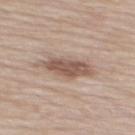Q: Was this lesion biopsied?
A: total-body-photography surveillance lesion; no biopsy
Q: Automated lesion metrics?
A: a lesion–skin lightness drop of about 12 and a normalized lesion–skin contrast near 8
Q: Lesion size?
A: ~5.5 mm (longest diameter)
Q: What are the patient's age and sex?
A: male, approximately 60 years of age
Q: Where on the body is the lesion?
A: the mid back
Q: What is the imaging modality?
A: 15 mm crop, total-body photography